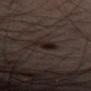Clinical impression:
The lesion was photographed on a routine skin check and not biopsied; there is no pathology result.
Clinical summary:
A 15 mm close-up extracted from a 3D total-body photography capture. The lesion is on the lower back. A male subject, aged 48 to 52. Measured at roughly 3.5 mm in maximum diameter. Captured under cross-polarized illumination.